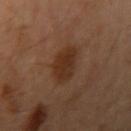biopsy_status: not biopsied; imaged during a skin examination
patient:
  sex: male
  age_approx: 50
site: left arm
lesion_size:
  long_diameter_mm_approx: 4.0
image:
  source: total-body photography crop
  field_of_view_mm: 15